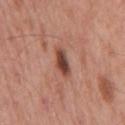notes=catalogued during a skin exam; not biopsied | lesion size=about 3.5 mm | body site=the mid back | subject=male, aged 53 to 57 | illumination=white-light | imaging modality=~15 mm crop, total-body skin-cancer survey.